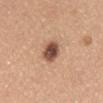Clinical impression:
The lesion was tiled from a total-body skin photograph and was not biopsied.
Clinical summary:
This is a white-light tile. A roughly 15 mm field-of-view crop from a total-body skin photograph. A female subject aged around 55. The lesion is located on the abdomen. Approximately 3 mm at its widest.